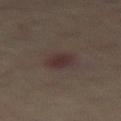The lesion was tiled from a total-body skin photograph and was not biopsied. This image is a 15 mm lesion crop taken from a total-body photograph. Located on the right thigh. A male patient about 60 years old.